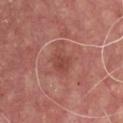Case summary:
• biopsy status — no biopsy performed (imaged during a skin exam)
• diameter — about 3 mm
• image source — total-body-photography crop, ~15 mm field of view
• patient — male, aged approximately 60
• location — the chest
• automated lesion analysis — a footprint of about 5.5 mm² and an outline eccentricity of about 0.7 (0 = round, 1 = elongated); a border-irregularity index near 3/10 and internal color variation of about 1.5 on a 0–10 scale; a nevus-likeness score of about 0/100
• lighting — white-light illumination